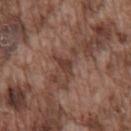{
  "biopsy_status": "not biopsied; imaged during a skin examination",
  "lighting": "white-light",
  "site": "chest",
  "patient": {
    "sex": "male",
    "age_approx": 75
  },
  "image": {
    "source": "total-body photography crop",
    "field_of_view_mm": 15
  },
  "lesion_size": {
    "long_diameter_mm_approx": 2.5
  }
}